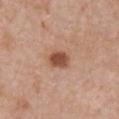Clinical impression:
The lesion was tiled from a total-body skin photograph and was not biopsied.
Acquisition and patient details:
The lesion is located on the chest. Longest diameter approximately 2.5 mm. A 15 mm close-up tile from a total-body photography series done for melanoma screening. A female patient approximately 40 years of age.